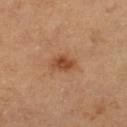Assessment: Recorded during total-body skin imaging; not selected for excision or biopsy. Acquisition and patient details: Captured under cross-polarized illumination. Cropped from a whole-body photographic skin survey; the tile spans about 15 mm. The lesion is on the left lower leg. A female subject roughly 60 years of age. Automated image analysis of the tile measured an area of roughly 5 mm², a shape eccentricity near 0.7, and two-axis asymmetry of about 0.2. The analysis additionally found an average lesion color of about L≈49 a*≈24 b*≈36 (CIELAB), about 11 CIELAB-L* units darker than the surrounding skin, and a lesion-to-skin contrast of about 8 (normalized; higher = more distinct). And it measured a border-irregularity rating of about 2/10, internal color variation of about 2.5 on a 0–10 scale, and radial color variation of about 1. And it measured a lesion-detection confidence of about 100/100.